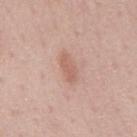workup: catalogued during a skin exam; not biopsied.